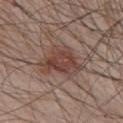Background:
The tile uses white-light illumination. Cropped from a total-body skin-imaging series; the visible field is about 15 mm. Automated image analysis of the tile measured a shape eccentricity near 0.65 and a symmetry-axis asymmetry near 0.45. The software also gave a lesion color around L≈43 a*≈19 b*≈23 in CIELAB, about 10 CIELAB-L* units darker than the surrounding skin, and a lesion-to-skin contrast of about 8 (normalized; higher = more distinct). The software also gave border irregularity of about 4.5 on a 0–10 scale and peripheral color asymmetry of about 1.5. The analysis additionally found a classifier nevus-likeness of about 65/100 and lesion-presence confidence of about 100/100. On the chest. A male subject, roughly 65 years of age. The recorded lesion diameter is about 5.5 mm.